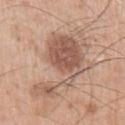A close-up tile cropped from a whole-body skin photograph, about 15 mm across.
The lesion is located on the left upper arm.
A male subject aged 53 to 57.